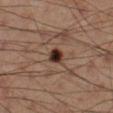Findings:
* workup · catalogued during a skin exam; not biopsied
* tile lighting · cross-polarized
* image-analysis metrics · a border-irregularity index near 2/10, a within-lesion color-variation index near 4/10, and peripheral color asymmetry of about 1; a nevus-likeness score of about 100/100 and a detector confidence of about 100 out of 100 that the crop contains a lesion
* body site · the right lower leg
* acquisition · total-body-photography crop, ~15 mm field of view
* subject · male, in their 50s